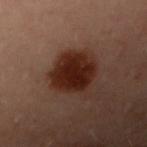Imaged during a routine full-body skin examination; the lesion was not biopsied and no histopathology is available. The recorded lesion diameter is about 4.5 mm. A 15 mm close-up extracted from a 3D total-body photography capture. From the left arm. Captured under cross-polarized illumination. A female subject aged 58–62.